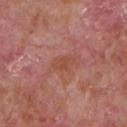Case summary:
- follow-up · imaged on a skin check; not biopsied
- patient · male, aged 63 to 67
- site · the chest
- automated metrics · a classifier nevus-likeness of about 0/100 and a lesion-detection confidence of about 100/100
- image source · ~15 mm crop, total-body skin-cancer survey
- lesion size · ≈3 mm